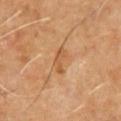Findings:
* biopsy status — imaged on a skin check; not biopsied
* tile lighting — cross-polarized
* subject — male, in their 60s
* location — the chest
* imaging modality — ~15 mm tile from a whole-body skin photo
* automated metrics — an area of roughly 3.5 mm², an eccentricity of roughly 0.9, and a shape-asymmetry score of about 0.45 (0 = symmetric); a classifier nevus-likeness of about 0/100 and lesion-presence confidence of about 100/100